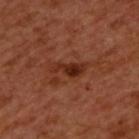A 15 mm close-up tile from a total-body photography series done for melanoma screening. Automated image analysis of the tile measured a lesion area of about 5.5 mm², an outline eccentricity of about 0.9 (0 = round, 1 = elongated), and a shape-asymmetry score of about 0.4 (0 = symmetric). Imaged with cross-polarized lighting. From the upper back. A male subject, roughly 50 years of age.